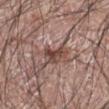| feature | finding |
|---|---|
| follow-up | total-body-photography surveillance lesion; no biopsy |
| acquisition | ~15 mm tile from a whole-body skin photo |
| lesion diameter | about 3 mm |
| tile lighting | white-light illumination |
| patient | male, in their 70s |
| automated lesion analysis | a lesion color around L≈44 a*≈19 b*≈23 in CIELAB and a lesion–skin lightness drop of about 12; border irregularity of about 4.5 on a 0–10 scale, internal color variation of about 4 on a 0–10 scale, and peripheral color asymmetry of about 1.5 |
| site | the left forearm |